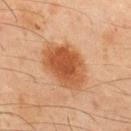Captured during whole-body skin photography for melanoma surveillance; the lesion was not biopsied. The lesion's longest dimension is about 6 mm. From the mid back. A roughly 15 mm field-of-view crop from a total-body skin photograph. The patient is a male approximately 45 years of age.